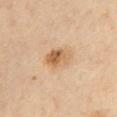workup: catalogued during a skin exam; not biopsied
subject: male, in their mid-60s
illumination: cross-polarized
lesion diameter: ~3.5 mm (longest diameter)
TBP lesion metrics: a lesion color around L≈62 a*≈19 b*≈37 in CIELAB, a lesion–skin lightness drop of about 11, and a lesion-to-skin contrast of about 7.5 (normalized; higher = more distinct); border irregularity of about 2 on a 0–10 scale, internal color variation of about 8.5 on a 0–10 scale, and peripheral color asymmetry of about 3; a nevus-likeness score of about 80/100
site: the chest
imaging modality: ~15 mm tile from a whole-body skin photo